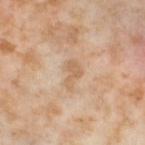The lesion was photographed on a routine skin check and not biopsied; there is no pathology result. Automated image analysis of the tile measured a lesion color around L≈63 a*≈18 b*≈34 in CIELAB, about 8 CIELAB-L* units darker than the surrounding skin, and a normalized lesion–skin contrast near 5.5. The analysis additionally found a border-irregularity rating of about 5.5/10, a within-lesion color-variation index near 1/10, and a peripheral color-asymmetry measure near 0.5. A female patient aged 53 to 57. A 15 mm close-up tile from a total-body photography series done for melanoma screening. Located on the left thigh. The tile uses cross-polarized illumination. The lesion's longest dimension is about 3.5 mm.